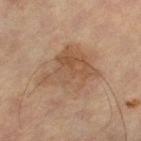- biopsy status · total-body-photography surveillance lesion; no biopsy
- image · 15 mm crop, total-body photography
- illumination · cross-polarized illumination
- automated metrics · a within-lesion color-variation index near 4/10 and radial color variation of about 1.5
- patient · male, aged 63 to 67
- lesion diameter · ~6.5 mm (longest diameter)
- body site · the left leg